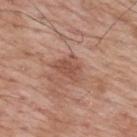biopsy_status: not biopsied; imaged during a skin examination
lesion_size:
  long_diameter_mm_approx: 3.5
image:
  source: total-body photography crop
  field_of_view_mm: 15
patient:
  sex: male
  age_approx: 70
site: upper back